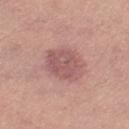follow-up — catalogued during a skin exam; not biopsied | lesion diameter — about 5 mm | patient — female, approximately 65 years of age | image source — ~15 mm tile from a whole-body skin photo | body site — the right thigh | TBP lesion metrics — an eccentricity of roughly 0.65 and a symmetry-axis asymmetry near 0.15; a mean CIELAB color near L≈55 a*≈23 b*≈21 and a lesion-to-skin contrast of about 6.5 (normalized; higher = more distinct); internal color variation of about 3.5 on a 0–10 scale and radial color variation of about 1.5; a nevus-likeness score of about 5/100 and a detector confidence of about 100 out of 100 that the crop contains a lesion | lighting — white-light illumination.